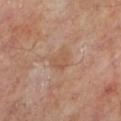Case summary:
- follow-up — catalogued during a skin exam; not biopsied
- lesion diameter — ≈2.5 mm
- site — the left lower leg
- imaging modality — ~15 mm tile from a whole-body skin photo
- subject — male, aged 48–52